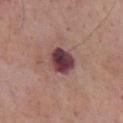The lesion was photographed on a routine skin check and not biopsied; there is no pathology result. The total-body-photography lesion software estimated a border-irregularity rating of about 1/10 and a within-lesion color-variation index near 9.5/10. This is a white-light tile. A 15 mm close-up tile from a total-body photography series done for melanoma screening. Longest diameter approximately 3.5 mm. The lesion is located on the chest. A male patient approximately 65 years of age.